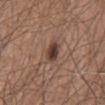follow-up — total-body-photography surveillance lesion; no biopsy
patient — male, aged 63 to 67
image — 15 mm crop, total-body photography
location — the abdomen
lighting — white-light illumination
TBP lesion metrics — a footprint of about 4.5 mm² and a shape-asymmetry score of about 0.15 (0 = symmetric)